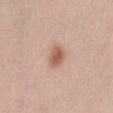<tbp_lesion>
<biopsy_status>not biopsied; imaged during a skin examination</biopsy_status>
<patient>
  <sex>female</sex>
  <age_approx>40</age_approx>
</patient>
<site>left thigh</site>
<lesion_size>
  <long_diameter_mm_approx>2.5</long_diameter_mm_approx>
</lesion_size>
<automated_metrics>
  <area_mm2_approx>5.0</area_mm2_approx>
  <eccentricity>0.3</eccentricity>
  <shape_asymmetry>0.2</shape_asymmetry>
  <border_irregularity_0_10>2.0</border_irregularity_0_10>
  <color_variation_0_10>3.0</color_variation_0_10>
  <nevus_likeness_0_100>95</nevus_likeness_0_100>
  <lesion_detection_confidence_0_100>100</lesion_detection_confidence_0_100>
</automated_metrics>
<image>
  <source>total-body photography crop</source>
  <field_of_view_mm>15</field_of_view_mm>
</image>
<lighting>white-light</lighting>
</tbp_lesion>